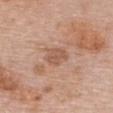Q: Was a biopsy performed?
A: catalogued during a skin exam; not biopsied
Q: Patient demographics?
A: female, roughly 60 years of age
Q: What is the imaging modality?
A: ~15 mm tile from a whole-body skin photo
Q: How large is the lesion?
A: ≈2.5 mm
Q: How was the tile lit?
A: white-light
Q: Where on the body is the lesion?
A: the chest